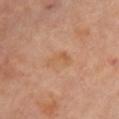The lesion was tiled from a total-body skin photograph and was not biopsied.